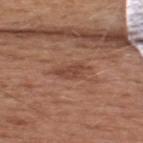Recorded during total-body skin imaging; not selected for excision or biopsy. A male subject, in their mid-50s. On the back. A region of skin cropped from a whole-body photographic capture, roughly 15 mm wide. Approximately 4 mm at its widest. The tile uses white-light illumination. An algorithmic analysis of the crop reported an area of roughly 5 mm², an eccentricity of roughly 0.9, and two-axis asymmetry of about 0.35. The software also gave a lesion color around L≈45 a*≈22 b*≈28 in CIELAB, about 8 CIELAB-L* units darker than the surrounding skin, and a normalized border contrast of about 6.5.